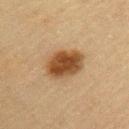<case>
<patient>
  <sex>female</sex>
  <age_approx>65</age_approx>
</patient>
<site>left upper arm</site>
<image>
  <source>total-body photography crop</source>
  <field_of_view_mm>15</field_of_view_mm>
</image>
</case>